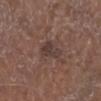Impression:
This lesion was catalogued during total-body skin photography and was not selected for biopsy.
Context:
A male patient, in their mid- to late 70s. On the left lower leg. This is a white-light tile. Automated image analysis of the tile measured an average lesion color of about L≈37 a*≈15 b*≈19 (CIELAB) and a lesion–skin lightness drop of about 7. The software also gave border irregularity of about 2.5 on a 0–10 scale and internal color variation of about 3 on a 0–10 scale. The lesion's longest dimension is about 3.5 mm. A 15 mm close-up extracted from a 3D total-body photography capture.